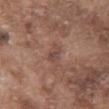The lesion was tiled from a total-body skin photograph and was not biopsied. Cropped from a whole-body photographic skin survey; the tile spans about 15 mm. The lesion is on the chest. The patient is a male in their mid- to late 70s.